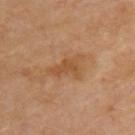The lesion was photographed on a routine skin check and not biopsied; there is no pathology result. A region of skin cropped from a whole-body photographic capture, roughly 15 mm wide. The lesion-visualizer software estimated an outline eccentricity of about 0.85 (0 = round, 1 = elongated) and two-axis asymmetry of about 0.45. The software also gave a lesion–skin lightness drop of about 7 and a normalized lesion–skin contrast near 6. The analysis additionally found a border-irregularity rating of about 5.5/10, a color-variation rating of about 2.5/10, and peripheral color asymmetry of about 1. The software also gave an automated nevus-likeness rating near 0 out of 100 and a lesion-detection confidence of about 100/100. From the upper back. This is a cross-polarized tile. Approximately 4.5 mm at its widest. A male subject in their 70s.